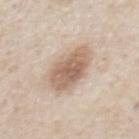Assessment: This lesion was catalogued during total-body skin photography and was not selected for biopsy. Clinical summary: Located on the chest. A close-up tile cropped from a whole-body skin photograph, about 15 mm across. A male subject, aged around 60.